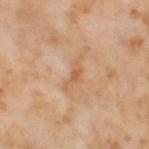Recorded during total-body skin imaging; not selected for excision or biopsy. The subject is a female in their mid- to late 50s. A 15 mm close-up extracted from a 3D total-body photography capture. On the left thigh. Automated image analysis of the tile measured an average lesion color of about L≈61 a*≈21 b*≈37 (CIELAB), a lesion–skin lightness drop of about 7, and a normalized border contrast of about 6. The tile uses cross-polarized illumination. The lesion's longest dimension is about 4 mm.